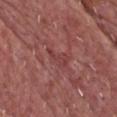The lesion was tiled from a total-body skin photograph and was not biopsied.
The lesion is on the front of the torso.
The tile uses white-light illumination.
A male subject aged around 70.
A roughly 15 mm field-of-view crop from a total-body skin photograph.
Longest diameter approximately 3.5 mm.